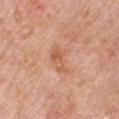Notes:
• follow-up · imaged on a skin check; not biopsied
• tile lighting · white-light illumination
• patient · male, in their 60s
• location · the arm
• image source · 15 mm crop, total-body photography